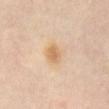follow-up=no biopsy performed (imaged during a skin exam) | imaging modality=total-body-photography crop, ~15 mm field of view | patient=female, approximately 60 years of age | anatomic site=the abdomen.